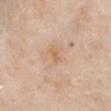Impression:
Recorded during total-body skin imaging; not selected for excision or biopsy.
Acquisition and patient details:
The total-body-photography lesion software estimated a symmetry-axis asymmetry near 0.4. The analysis additionally found a mean CIELAB color near L≈63 a*≈18 b*≈35, about 7 CIELAB-L* units darker than the surrounding skin, and a normalized lesion–skin contrast near 5.5. And it measured a border-irregularity index near 3.5/10, a within-lesion color-variation index near 0/10, and radial color variation of about 0. And it measured a nevus-likeness score of about 0/100. A 15 mm close-up tile from a total-body photography series done for melanoma screening. A female subject approximately 65 years of age. The lesion is on the chest. Longest diameter approximately 2 mm.